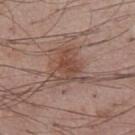{
  "biopsy_status": "not biopsied; imaged during a skin examination",
  "site": "chest",
  "image": {
    "source": "total-body photography crop",
    "field_of_view_mm": 15
  },
  "patient": {
    "sex": "male",
    "age_approx": 55
  }
}